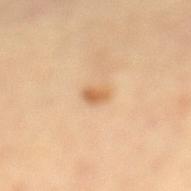| key | value |
|---|---|
| notes | total-body-photography surveillance lesion; no biopsy |
| image source | ~15 mm tile from a whole-body skin photo |
| automated lesion analysis | a lesion area of about 3 mm², an eccentricity of roughly 0.75, and a shape-asymmetry score of about 0.3 (0 = symmetric); a lesion color around L≈64 a*≈20 b*≈40 in CIELAB, roughly 11 lightness units darker than nearby skin, and a normalized border contrast of about 7; a border-irregularity rating of about 2.5/10, a within-lesion color-variation index near 2.5/10, and radial color variation of about 1; a nevus-likeness score of about 85/100 and a detector confidence of about 100 out of 100 that the crop contains a lesion |
| location | the mid back |
| lesion diameter | ≈2.5 mm |
| patient | female, aged 63–67 |